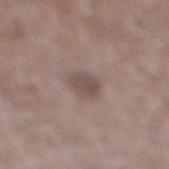This lesion was catalogued during total-body skin photography and was not selected for biopsy. The subject is a male aged 68–72. A 15 mm crop from a total-body photograph taken for skin-cancer surveillance. The lesion is located on the right lower leg. Imaged with white-light lighting. About 2.5 mm across.